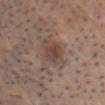biopsy status = no biopsy performed (imaged during a skin exam) | site = the head or neck | subject = male, aged 53 to 57 | imaging modality = 15 mm crop, total-body photography.